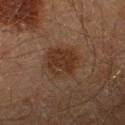Clinical impression: The lesion was tiled from a total-body skin photograph and was not biopsied. Background: The recorded lesion diameter is about 5 mm. A male subject, in their 60s. The lesion is located on the right lower leg. A 15 mm crop from a total-body photograph taken for skin-cancer surveillance. The lesion-visualizer software estimated a lesion area of about 12 mm² and a symmetry-axis asymmetry near 0.2. It also reported a color-variation rating of about 2.5/10 and peripheral color asymmetry of about 1.